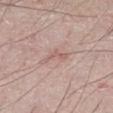Impression:
Imaged during a routine full-body skin examination; the lesion was not biopsied and no histopathology is available.
Background:
The tile uses white-light illumination. The subject is a male in their mid- to late 80s or older. This image is a 15 mm lesion crop taken from a total-body photograph. The lesion is located on the front of the torso. Automated tile analysis of the lesion measured a lesion area of about 3 mm², an eccentricity of roughly 0.9, and two-axis asymmetry of about 0.55. It also reported an average lesion color of about L≈59 a*≈19 b*≈23 (CIELAB) and about 7 CIELAB-L* units darker than the surrounding skin. About 3 mm across.